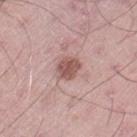The lesion was tiled from a total-body skin photograph and was not biopsied. The lesion is on the right thigh. A male patient, roughly 45 years of age. A roughly 15 mm field-of-view crop from a total-body skin photograph. Captured under white-light illumination.